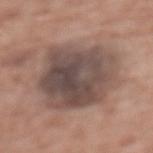{"biopsy_status": "not biopsied; imaged during a skin examination"}